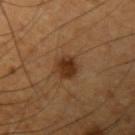biopsy status: catalogued during a skin exam; not biopsied
lesion size: about 3 mm
subject: male, aged 63 to 67
image: ~15 mm crop, total-body skin-cancer survey
anatomic site: the left upper arm
automated metrics: an area of roughly 6 mm², an outline eccentricity of about 0.4 (0 = round, 1 = elongated), and two-axis asymmetry of about 0.2; a lesion color around L≈32 a*≈20 b*≈31 in CIELAB, a lesion–skin lightness drop of about 11, and a normalized border contrast of about 10